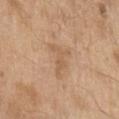Clinical impression:
The lesion was tiled from a total-body skin photograph and was not biopsied.
Background:
A male patient, aged 68 to 72. A 15 mm close-up extracted from a 3D total-body photography capture. From the left upper arm.